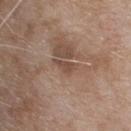Clinical impression:
The lesion was tiled from a total-body skin photograph and was not biopsied.
Context:
The patient is a female roughly 75 years of age. Located on the upper back. Approximately 2.5 mm at its widest. The lesion-visualizer software estimated an eccentricity of roughly 0.9 and a symmetry-axis asymmetry near 0.5. It also reported about 9 CIELAB-L* units darker than the surrounding skin and a normalized lesion–skin contrast near 7. The analysis additionally found a nevus-likeness score of about 0/100. Captured under white-light illumination. A region of skin cropped from a whole-body photographic capture, roughly 15 mm wide.